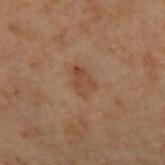Q: Was this lesion biopsied?
A: catalogued during a skin exam; not biopsied
Q: Where on the body is the lesion?
A: the left arm
Q: Illumination type?
A: cross-polarized illumination
Q: How was this image acquired?
A: total-body-photography crop, ~15 mm field of view
Q: What are the patient's age and sex?
A: female, aged 53 to 57
Q: What did automated image analysis measure?
A: an average lesion color of about L≈37 a*≈17 b*≈27 (CIELAB), a lesion–skin lightness drop of about 6, and a normalized lesion–skin contrast near 6